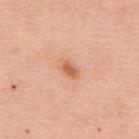Imaged during a routine full-body skin examination; the lesion was not biopsied and no histopathology is available.
Approximately 2.5 mm at its widest.
The patient is a female in their mid- to late 60s.
Cropped from a total-body skin-imaging series; the visible field is about 15 mm.
On the upper back.